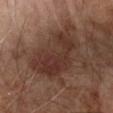follow-up: catalogued during a skin exam; not biopsied
subject: male, in their mid-60s
tile lighting: cross-polarized
imaging modality: ~15 mm tile from a whole-body skin photo
location: the right forearm
lesion size: ≈9.5 mm